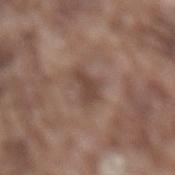• notes — total-body-photography surveillance lesion; no biopsy
• subject — male, aged 73 to 77
• imaging modality — 15 mm crop, total-body photography
• lesion size — ~4 mm (longest diameter)
• automated metrics — a footprint of about 6 mm² and an outline eccentricity of about 0.9 (0 = round, 1 = elongated); a lesion color around L≈44 a*≈17 b*≈23 in CIELAB, roughly 9 lightness units darker than nearby skin, and a lesion-to-skin contrast of about 7 (normalized; higher = more distinct)
• tile lighting — white-light illumination
• body site — the lower back